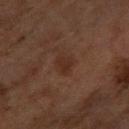Notes:
• workup: total-body-photography surveillance lesion; no biopsy
• size: ≈2.5 mm
• body site: the right forearm
• imaging modality: ~15 mm tile from a whole-body skin photo
• subject: female, aged approximately 60
• tile lighting: cross-polarized
• automated lesion analysis: a footprint of about 4 mm², an outline eccentricity of about 0.6 (0 = round, 1 = elongated), and a shape-asymmetry score of about 0.4 (0 = symmetric); a lesion–skin lightness drop of about 5; a border-irregularity index near 3.5/10, a within-lesion color-variation index near 1.5/10, and peripheral color asymmetry of about 0.5; a classifier nevus-likeness of about 5/100 and a detector confidence of about 100 out of 100 that the crop contains a lesion